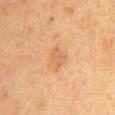The lesion is on the mid back. A female patient aged approximately 60. A 15 mm close-up extracted from a 3D total-body photography capture.